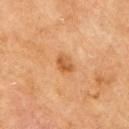Q: What is the lesion's diameter?
A: ~2.5 mm (longest diameter)
Q: Patient demographics?
A: male, approximately 70 years of age
Q: What is the imaging modality?
A: ~15 mm crop, total-body skin-cancer survey
Q: How was the tile lit?
A: cross-polarized
Q: What is the anatomic site?
A: the arm